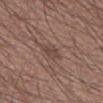{
  "biopsy_status": "not biopsied; imaged during a skin examination",
  "automated_metrics": {
    "area_mm2_approx": 4.5,
    "shape_asymmetry": 0.2,
    "vs_skin_darker_L": 7.0,
    "vs_skin_contrast_norm": 5.5,
    "color_variation_0_10": 2.0,
    "peripheral_color_asymmetry": 0.5,
    "nevus_likeness_0_100": 0,
    "lesion_detection_confidence_0_100": 100
  },
  "patient": {
    "sex": "male",
    "age_approx": 55
  },
  "lesion_size": {
    "long_diameter_mm_approx": 2.5
  },
  "lighting": "white-light",
  "image": {
    "source": "total-body photography crop",
    "field_of_view_mm": 15
  },
  "site": "right forearm"
}